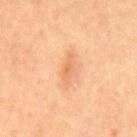{"biopsy_status": "not biopsied; imaged during a skin examination", "lighting": "cross-polarized", "automated_metrics": {"area_mm2_approx": 3.0, "eccentricity": 0.95, "vs_skin_darker_L": 8.0, "border_irregularity_0_10": 3.5, "color_variation_0_10": 0.5, "peripheral_color_asymmetry": 0.0, "nevus_likeness_0_100": 0, "lesion_detection_confidence_0_100": 100}, "image": {"source": "total-body photography crop", "field_of_view_mm": 15}, "patient": {"sex": "male", "age_approx": 55}, "site": "back"}